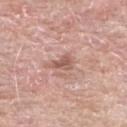biopsy status=imaged on a skin check; not biopsied | subject=male, about 65 years old | image=total-body-photography crop, ~15 mm field of view | site=the upper back | lesion size=≈2.5 mm.